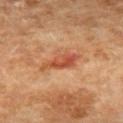No biopsy was performed on this lesion — it was imaged during a full skin examination and was not determined to be concerning. The recorded lesion diameter is about 5 mm. A roughly 15 mm field-of-view crop from a total-body skin photograph. The tile uses cross-polarized illumination. Located on the upper back. The patient is a female aged approximately 60.